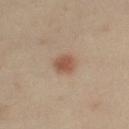Impression: Recorded during total-body skin imaging; not selected for excision or biopsy. Background: A 15 mm close-up extracted from a 3D total-body photography capture. Located on the left arm. A female patient, approximately 40 years of age. This is a cross-polarized tile. The recorded lesion diameter is about 3 mm.